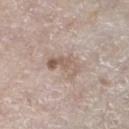notes: total-body-photography surveillance lesion; no biopsy | anatomic site: the left lower leg | illumination: white-light | size: ~4.5 mm (longest diameter) | acquisition: 15 mm crop, total-body photography | subject: female, in their mid- to late 70s | TBP lesion metrics: an outline eccentricity of about 0.85 (0 = round, 1 = elongated) and a symmetry-axis asymmetry near 0.45; a lesion–skin lightness drop of about 10 and a normalized border contrast of about 7.